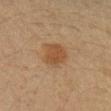follow-up: total-body-photography surveillance lesion; no biopsy | image: ~15 mm crop, total-body skin-cancer survey | patient: female, aged 38 to 42 | body site: the left upper arm | illumination: cross-polarized | size: about 4 mm.